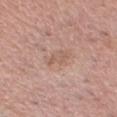biopsy status: total-body-photography surveillance lesion; no biopsy
lesion diameter: ≈2.5 mm
lighting: white-light illumination
imaging modality: ~15 mm tile from a whole-body skin photo
anatomic site: the head or neck
subject: male, about 60 years old